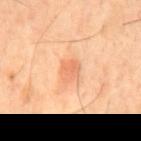Acquisition and patient details:
This is a cross-polarized tile. A 15 mm close-up extracted from a 3D total-body photography capture. The recorded lesion diameter is about 2.5 mm. From the mid back. The subject is a male aged approximately 45.